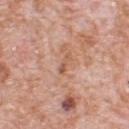Clinical impression:
Imaged during a routine full-body skin examination; the lesion was not biopsied and no histopathology is available.
Image and clinical context:
Located on the back. Automated tile analysis of the lesion measured roughly 8 lightness units darker than nearby skin. And it measured border irregularity of about 3.5 on a 0–10 scale and a peripheral color-asymmetry measure near 0. The analysis additionally found a nevus-likeness score of about 0/100 and a detector confidence of about 100 out of 100 that the crop contains a lesion. Captured under white-light illumination. A lesion tile, about 15 mm wide, cut from a 3D total-body photograph. A male subject, aged around 80.